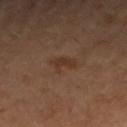Captured during whole-body skin photography for melanoma surveillance; the lesion was not biopsied.
The lesion's longest dimension is about 3 mm.
A close-up tile cropped from a whole-body skin photograph, about 15 mm across.
Automated image analysis of the tile measured a footprint of about 4.5 mm². And it measured a lesion–skin lightness drop of about 6 and a normalized lesion–skin contrast near 6.5. And it measured border irregularity of about 4.5 on a 0–10 scale, a color-variation rating of about 1.5/10, and peripheral color asymmetry of about 0.5. It also reported a nevus-likeness score of about 0/100 and a lesion-detection confidence of about 100/100.
Located on the right forearm.
A female patient, in their 60s.
This is a cross-polarized tile.